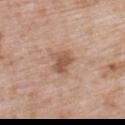Recorded during total-body skin imaging; not selected for excision or biopsy.
About 3 mm across.
A female subject, aged approximately 70.
From the left upper arm.
An algorithmic analysis of the crop reported an average lesion color of about L≈55 a*≈20 b*≈30 (CIELAB), a lesion–skin lightness drop of about 10, and a lesion-to-skin contrast of about 7 (normalized; higher = more distinct). And it measured border irregularity of about 3 on a 0–10 scale, a within-lesion color-variation index near 3/10, and peripheral color asymmetry of about 1.
A 15 mm close-up extracted from a 3D total-body photography capture.
Captured under white-light illumination.